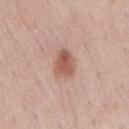| key | value |
|---|---|
| notes | imaged on a skin check; not biopsied |
| size | ≈3 mm |
| tile lighting | white-light |
| patient | male, aged 73–77 |
| automated lesion analysis | a border-irregularity rating of about 2/10, a color-variation rating of about 4/10, and peripheral color asymmetry of about 1.5; a detector confidence of about 100 out of 100 that the crop contains a lesion |
| site | the right thigh |
| imaging modality | ~15 mm tile from a whole-body skin photo |